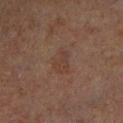{"biopsy_status": "not biopsied; imaged during a skin examination", "lesion_size": {"long_diameter_mm_approx": 3.5}, "lighting": "cross-polarized", "automated_metrics": {"area_mm2_approx": 6.0, "eccentricity": 0.8, "shape_asymmetry": 0.2, "border_irregularity_0_10": 2.0, "peripheral_color_asymmetry": 0.5}, "site": "right lower leg", "patient": {"sex": "male", "age_approx": 65}, "image": {"source": "total-body photography crop", "field_of_view_mm": 15}}